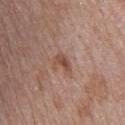patient: male, in their 50s | location: the back | size: ~3 mm (longest diameter) | acquisition: 15 mm crop, total-body photography | automated lesion analysis: a footprint of about 4.5 mm², an eccentricity of roughly 0.65, and a shape-asymmetry score of about 0.45 (0 = symmetric); a peripheral color-asymmetry measure near 1 | tile lighting: white-light.